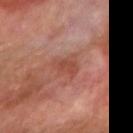Captured during whole-body skin photography for melanoma surveillance; the lesion was not biopsied.